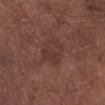Imaged during a routine full-body skin examination; the lesion was not biopsied and no histopathology is available. The lesion-visualizer software estimated a lesion area of about 7.5 mm², an outline eccentricity of about 0.6 (0 = round, 1 = elongated), and a shape-asymmetry score of about 0.55 (0 = symmetric). And it measured a lesion–skin lightness drop of about 5 and a normalized border contrast of about 5.5. And it measured border irregularity of about 7.5 on a 0–10 scale and peripheral color asymmetry of about 0.5. A male patient, approximately 55 years of age. Measured at roughly 3.5 mm in maximum diameter. A lesion tile, about 15 mm wide, cut from a 3D total-body photograph. The tile uses white-light illumination. The lesion is on the right lower leg.